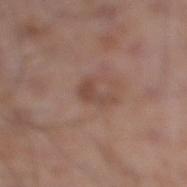The lesion was tiled from a total-body skin photograph and was not biopsied.
A male subject about 55 years old.
From the leg.
An algorithmic analysis of the crop reported a lesion area of about 3.5 mm², an outline eccentricity of about 0.8 (0 = round, 1 = elongated), and a shape-asymmetry score of about 0.6 (0 = symmetric).
A 15 mm close-up extracted from a 3D total-body photography capture.